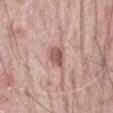Notes:
– follow-up: imaged on a skin check; not biopsied
– lesion size: ~3 mm (longest diameter)
– patient: male, aged 78 to 82
– anatomic site: the abdomen
– image: ~15 mm tile from a whole-body skin photo